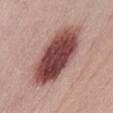workup: imaged on a skin check; not biopsied
image source: ~15 mm crop, total-body skin-cancer survey
body site: the abdomen
subject: female, in their 50s